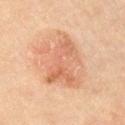The lesion was tiled from a total-body skin photograph and was not biopsied. From the arm. A male subject, roughly 60 years of age. A close-up tile cropped from a whole-body skin photograph, about 15 mm across.